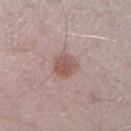biopsy_status: not biopsied; imaged during a skin examination
site: right lower leg
patient:
  sex: male
  age_approx: 40
image:
  source: total-body photography crop
  field_of_view_mm: 15